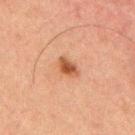  biopsy_status: not biopsied; imaged during a skin examination
  image:
    source: total-body photography crop
    field_of_view_mm: 15
  patient:
    sex: male
    age_approx: 50
  site: upper back
  lesion_size:
    long_diameter_mm_approx: 3.0